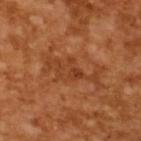Q: Is there a histopathology result?
A: no biopsy performed (imaged during a skin exam)
Q: What is the imaging modality?
A: ~15 mm crop, total-body skin-cancer survey
Q: What is the lesion's diameter?
A: ~7.5 mm (longest diameter)
Q: What are the patient's age and sex?
A: male, approximately 65 years of age